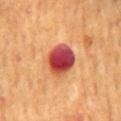Imaged during a routine full-body skin examination; the lesion was not biopsied and no histopathology is available. Measured at roughly 4 mm in maximum diameter. A close-up tile cropped from a whole-body skin photograph, about 15 mm across. A male subject aged around 70. The lesion is on the mid back. Automated tile analysis of the lesion measured a mean CIELAB color near L≈47 a*≈38 b*≈31, about 20 CIELAB-L* units darker than the surrounding skin, and a normalized border contrast of about 14.5. The software also gave a border-irregularity rating of about 1.5/10, a within-lesion color-variation index near 10/10, and peripheral color asymmetry of about 4. The analysis additionally found a classifier nevus-likeness of about 0/100.